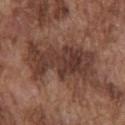The lesion was tiled from a total-body skin photograph and was not biopsied. From the front of the torso. Imaged with white-light lighting. Approximately 9 mm at its widest. A male subject, aged 73–77. A 15 mm close-up tile from a total-body photography series done for melanoma screening.